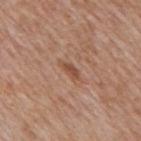Captured during whole-body skin photography for melanoma surveillance; the lesion was not biopsied.
The lesion is located on the back.
Measured at roughly 3 mm in maximum diameter.
An algorithmic analysis of the crop reported an area of roughly 3 mm² and a shape-asymmetry score of about 0.35 (0 = symmetric). The analysis additionally found a within-lesion color-variation index near 0/10 and a peripheral color-asymmetry measure near 0. The analysis additionally found a classifier nevus-likeness of about 0/100.
The patient is a male in their 60s.
This image is a 15 mm lesion crop taken from a total-body photograph.
This is a white-light tile.